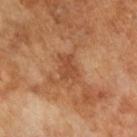Approximately 3.5 mm at its widest. The patient is a male aged 63 to 67. The total-body-photography lesion software estimated an automated nevus-likeness rating near 0 out of 100 and a lesion-detection confidence of about 100/100. A region of skin cropped from a whole-body photographic capture, roughly 15 mm wide.